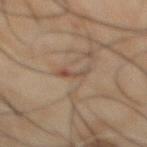Impression: Imaged during a routine full-body skin examination; the lesion was not biopsied and no histopathology is available. Acquisition and patient details: A 15 mm close-up tile from a total-body photography series done for melanoma screening. Captured under cross-polarized illumination. The patient is a male aged around 50. Automated tile analysis of the lesion measured an outline eccentricity of about 0.95 (0 = round, 1 = elongated) and two-axis asymmetry of about 0.5. The analysis additionally found a mean CIELAB color near L≈36 a*≈13 b*≈21, about 6 CIELAB-L* units darker than the surrounding skin, and a lesion-to-skin contrast of about 5.5 (normalized; higher = more distinct). It also reported a classifier nevus-likeness of about 0/100. Located on the abdomen.